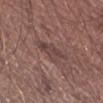Recorded during total-body skin imaging; not selected for excision or biopsy.
The recorded lesion diameter is about 4 mm.
A male patient, in their mid-60s.
A 15 mm crop from a total-body photograph taken for skin-cancer surveillance.
Automated image analysis of the tile measured border irregularity of about 5 on a 0–10 scale and a within-lesion color-variation index near 3/10. The analysis additionally found an automated nevus-likeness rating near 0 out of 100 and a lesion-detection confidence of about 60/100.
This is a white-light tile.
The lesion is on the right upper arm.